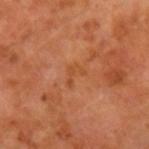No biopsy was performed on this lesion — it was imaged during a full skin examination and was not determined to be concerning.
About 2.5 mm across.
An algorithmic analysis of the crop reported a border-irregularity rating of about 7.5/10, a within-lesion color-variation index near 0/10, and radial color variation of about 0.
From the left forearm.
The tile uses cross-polarized illumination.
A male subject approximately 60 years of age.
A roughly 15 mm field-of-view crop from a total-body skin photograph.